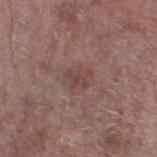workup: total-body-photography surveillance lesion; no biopsy | automated lesion analysis: a footprint of about 6.5 mm², a shape eccentricity near 0.6, and a shape-asymmetry score of about 0.25 (0 = symmetric) | location: the leg | image source: ~15 mm crop, total-body skin-cancer survey | diameter: ≈3 mm | subject: male, roughly 70 years of age.